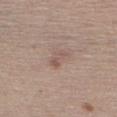The lesion was photographed on a routine skin check and not biopsied; there is no pathology result. Automated image analysis of the tile measured a footprint of about 3.5 mm² and an outline eccentricity of about 0.85 (0 = round, 1 = elongated). It also reported a lesion color around L≈54 a*≈17 b*≈23 in CIELAB, roughly 7 lightness units darker than nearby skin, and a normalized lesion–skin contrast near 5. The analysis additionally found a color-variation rating of about 2/10 and radial color variation of about 0.5. The software also gave a nevus-likeness score of about 0/100 and lesion-presence confidence of about 100/100. Longest diameter approximately 2.5 mm. On the right upper arm. A close-up tile cropped from a whole-body skin photograph, about 15 mm across. A male patient in their mid- to late 50s.